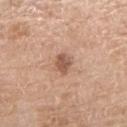Part of a total-body skin-imaging series; this lesion was reviewed on a skin check and was not flagged for biopsy. A lesion tile, about 15 mm wide, cut from a 3D total-body photograph. A male subject, approximately 70 years of age. The lesion is located on the right upper arm.